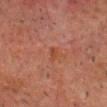A male patient, aged approximately 65.
This image is a 15 mm lesion crop taken from a total-body photograph.
This is a cross-polarized tile.
An algorithmic analysis of the crop reported a classifier nevus-likeness of about 0/100 and a lesion-detection confidence of about 100/100.
The lesion is on the head or neck.
Longest diameter approximately 1.5 mm.